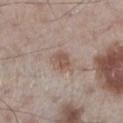Part of a total-body skin-imaging series; this lesion was reviewed on a skin check and was not flagged for biopsy.